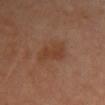Q: Was a biopsy performed?
A: no biopsy performed (imaged during a skin exam)
Q: Automated lesion metrics?
A: a lesion area of about 8 mm² and a shape-asymmetry score of about 0.25 (0 = symmetric); a lesion color around L≈41 a*≈21 b*≈31 in CIELAB, about 6 CIELAB-L* units darker than the surrounding skin, and a lesion-to-skin contrast of about 6 (normalized; higher = more distinct)
Q: How was this image acquired?
A: total-body-photography crop, ~15 mm field of view
Q: What are the patient's age and sex?
A: female, approximately 50 years of age
Q: Lesion location?
A: the chest